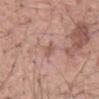subject: male, about 55 years old | site: the mid back | imaging modality: total-body-photography crop, ~15 mm field of view.